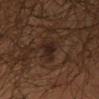biopsy status: catalogued during a skin exam; not biopsied
image-analysis metrics: a lesion area of about 4.5 mm², a shape eccentricity near 0.7, and a shape-asymmetry score of about 0.45 (0 = symmetric); an average lesion color of about L≈19 a*≈13 b*≈18 (CIELAB), about 7 CIELAB-L* units darker than the surrounding skin, and a lesion-to-skin contrast of about 10 (normalized; higher = more distinct); border irregularity of about 4.5 on a 0–10 scale, internal color variation of about 1.5 on a 0–10 scale, and peripheral color asymmetry of about 0.5; a classifier nevus-likeness of about 55/100 and lesion-presence confidence of about 95/100
anatomic site: the right upper arm
image source: 15 mm crop, total-body photography
size: ~3 mm (longest diameter)
subject: male, aged 33 to 37
illumination: cross-polarized